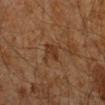The lesion was tiled from a total-body skin photograph and was not biopsied. A male subject, aged approximately 45. On the arm. Captured under cross-polarized illumination. A lesion tile, about 15 mm wide, cut from a 3D total-body photograph.